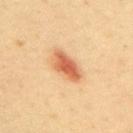Imaged during a routine full-body skin examination; the lesion was not biopsied and no histopathology is available.
This is a cross-polarized tile.
Located on the upper back.
The total-body-photography lesion software estimated border irregularity of about 2.5 on a 0–10 scale, internal color variation of about 4 on a 0–10 scale, and radial color variation of about 1. The software also gave an automated nevus-likeness rating near 100 out of 100 and a detector confidence of about 100 out of 100 that the crop contains a lesion.
The lesion's longest dimension is about 4.5 mm.
A region of skin cropped from a whole-body photographic capture, roughly 15 mm wide.
The subject is a male about 40 years old.